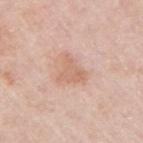Assessment: Recorded during total-body skin imaging; not selected for excision or biopsy. Clinical summary: The lesion is located on the right upper arm. This image is a 15 mm lesion crop taken from a total-body photograph. A female patient, in their mid-60s.